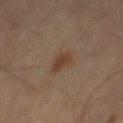| field | value |
|---|---|
| follow-up | total-body-photography surveillance lesion; no biopsy |
| location | the mid back |
| tile lighting | cross-polarized |
| patient | male, aged around 70 |
| acquisition | total-body-photography crop, ~15 mm field of view |
| diameter | about 3 mm |